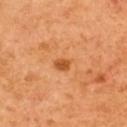Impression: The lesion was tiled from a total-body skin photograph and was not biopsied. Image and clinical context: The patient is a male aged 58 to 62. A 15 mm close-up tile from a total-body photography series done for melanoma screening. From the upper back. This is a cross-polarized tile.